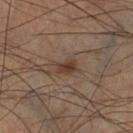No biopsy was performed on this lesion — it was imaged during a full skin examination and was not determined to be concerning. The tile uses cross-polarized illumination. The lesion-visualizer software estimated an area of roughly 4.5 mm², a shape eccentricity near 0.8, and a symmetry-axis asymmetry near 0.3. And it measured a lesion color around L≈36 a*≈16 b*≈24 in CIELAB, roughly 9 lightness units darker than nearby skin, and a normalized border contrast of about 8. The recorded lesion diameter is about 3 mm. Cropped from a total-body skin-imaging series; the visible field is about 15 mm. The lesion is located on the left lower leg. A male subject aged around 60.